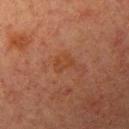notes — catalogued during a skin exam; not biopsied | illumination — cross-polarized illumination | site — the arm | patient — female, about 55 years old | image — ~15 mm crop, total-body skin-cancer survey | lesion diameter — about 2.5 mm.